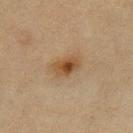Q: Is there a histopathology result?
A: total-body-photography surveillance lesion; no biopsy
Q: How was the tile lit?
A: cross-polarized illumination
Q: Patient demographics?
A: female, aged approximately 55
Q: What is the imaging modality?
A: ~15 mm crop, total-body skin-cancer survey
Q: What is the anatomic site?
A: the right thigh